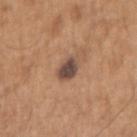Captured during whole-body skin photography for melanoma surveillance; the lesion was not biopsied. Located on the right upper arm. This image is a 15 mm lesion crop taken from a total-body photograph. A male patient, aged 63–67.